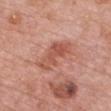  biopsy_status: not biopsied; imaged during a skin examination
  patient:
    sex: female
    age_approx: 60
  image:
    source: total-body photography crop
    field_of_view_mm: 15
  site: chest
  automated_metrics:
    cielab_L: 54
    cielab_a: 27
    cielab_b: 30
    vs_skin_darker_L: 9.0
    vs_skin_contrast_norm: 6.5
    border_irregularity_0_10: 5.0
    color_variation_0_10: 4.5
    peripheral_color_asymmetry: 1.5